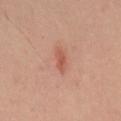{"biopsy_status": "not biopsied; imaged during a skin examination", "patient": {"sex": "male", "age_approx": 45}, "automated_metrics": {"vs_skin_darker_L": 8.0}, "site": "mid back", "image": {"source": "total-body photography crop", "field_of_view_mm": 15}, "lighting": "cross-polarized", "lesion_size": {"long_diameter_mm_approx": 3.0}}